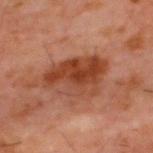This image is a 15 mm lesion crop taken from a total-body photograph. Located on the upper back. The patient is a male about 60 years old.